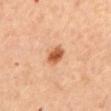Case summary:
– biopsy status · imaged on a skin check; not biopsied
– lesion diameter · ≈3 mm
– patient · female, aged 43–47
– image source · ~15 mm crop, total-body skin-cancer survey
– body site · the mid back